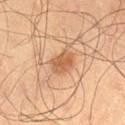Impression:
This lesion was catalogued during total-body skin photography and was not selected for biopsy.
Background:
Cropped from a total-body skin-imaging series; the visible field is about 15 mm. A male subject aged approximately 70. The lesion is on the left thigh.